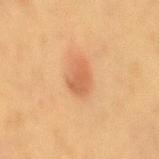Assessment:
Part of a total-body skin-imaging series; this lesion was reviewed on a skin check and was not flagged for biopsy.
Background:
Approximately 3 mm at its widest. Cropped from a whole-body photographic skin survey; the tile spans about 15 mm. A female subject, about 40 years old. Located on the mid back. The total-body-photography lesion software estimated an average lesion color of about L≈52 a*≈22 b*≈35 (CIELAB) and about 9 CIELAB-L* units darker than the surrounding skin. And it measured border irregularity of about 3 on a 0–10 scale and a within-lesion color-variation index near 2/10. This is a cross-polarized tile.